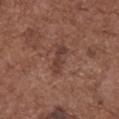Case summary:
- workup: imaged on a skin check; not biopsied
- anatomic site: the upper back
- acquisition: ~15 mm crop, total-body skin-cancer survey
- patient: female, aged approximately 75